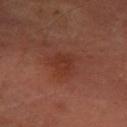The lesion was photographed on a routine skin check and not biopsied; there is no pathology result. A male subject in their 60s. Located on the left forearm. The total-body-photography lesion software estimated an average lesion color of about L≈34 a*≈25 b*≈28 (CIELAB), roughly 5 lightness units darker than nearby skin, and a normalized lesion–skin contrast near 5. And it measured a nevus-likeness score of about 40/100 and a detector confidence of about 100 out of 100 that the crop contains a lesion. Imaged with cross-polarized lighting. A roughly 15 mm field-of-view crop from a total-body skin photograph. The recorded lesion diameter is about 3.5 mm.